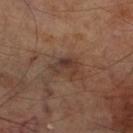| field | value |
|---|---|
| biopsy status | imaged on a skin check; not biopsied |
| body site | the leg |
| diameter | ≈3 mm |
| patient | male, in their 70s |
| imaging modality | total-body-photography crop, ~15 mm field of view |
| tile lighting | cross-polarized illumination |
| TBP lesion metrics | a footprint of about 5 mm², a shape eccentricity near 0.7, and two-axis asymmetry of about 0.4; a lesion color around L≈35 a*≈17 b*≈23 in CIELAB and a normalized border contrast of about 7; border irregularity of about 5 on a 0–10 scale, a within-lesion color-variation index near 4.5/10, and a peripheral color-asymmetry measure near 1 |